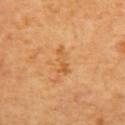biopsy status: no biopsy performed (imaged during a skin exam)
size: ≈3.5 mm
image: total-body-photography crop, ~15 mm field of view
automated lesion analysis: an area of roughly 4 mm², an outline eccentricity of about 0.9 (0 = round, 1 = elongated), and a symmetry-axis asymmetry near 0.35; an automated nevus-likeness rating near 0 out of 100 and a lesion-detection confidence of about 100/100
tile lighting: cross-polarized
patient: female
location: the back